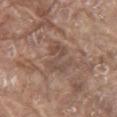Recorded during total-body skin imaging; not selected for excision or biopsy.
On the abdomen.
The subject is a male aged approximately 80.
The lesion's longest dimension is about 4 mm.
Captured under white-light illumination.
A 15 mm close-up extracted from a 3D total-body photography capture.